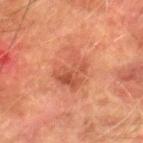Assessment:
Captured during whole-body skin photography for melanoma surveillance; the lesion was not biopsied.